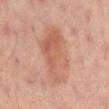• follow-up: catalogued during a skin exam; not biopsied
• image source: 15 mm crop, total-body photography
• size: ≈8 mm
• body site: the mid back
• automated metrics: an area of roughly 24 mm², a shape eccentricity near 0.9, and two-axis asymmetry of about 0.25; a classifier nevus-likeness of about 45/100 and a detector confidence of about 100 out of 100 that the crop contains a lesion
• subject: male, approximately 60 years of age
• tile lighting: cross-polarized illumination